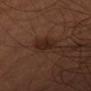Clinical summary:
A roughly 15 mm field-of-view crop from a total-body skin photograph. The lesion's longest dimension is about 2.5 mm. The lesion is on the right thigh. A male subject aged 48 to 52. An algorithmic analysis of the crop reported an area of roughly 3.5 mm², a shape eccentricity near 0.75, and a symmetry-axis asymmetry near 0.3. It also reported a mean CIELAB color near L≈22 a*≈18 b*≈22, roughly 7 lightness units darker than nearby skin, and a normalized lesion–skin contrast near 8. And it measured a border-irregularity rating of about 3/10, internal color variation of about 2 on a 0–10 scale, and a peripheral color-asymmetry measure near 0.5.